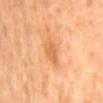The tile uses cross-polarized illumination. Approximately 4 mm at its widest. Automated image analysis of the tile measured an area of roughly 5.5 mm², an eccentricity of roughly 0.9, and a symmetry-axis asymmetry near 0.3. And it measured an average lesion color of about L≈64 a*≈27 b*≈42 (CIELAB) and a normalized lesion–skin contrast near 6. A roughly 15 mm field-of-view crop from a total-body skin photograph. A female patient roughly 50 years of age. The lesion is on the mid back.